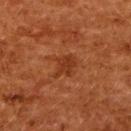workup = catalogued during a skin exam; not biopsied
TBP lesion metrics = a lesion area of about 5.5 mm²
image source = ~15 mm tile from a whole-body skin photo
body site = the upper back
subject = female, about 50 years old
lighting = cross-polarized
lesion size = ≈3 mm